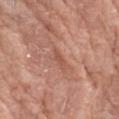Captured during whole-body skin photography for melanoma surveillance; the lesion was not biopsied. Automated tile analysis of the lesion measured a footprint of about 2.5 mm². It also reported about 7 CIELAB-L* units darker than the surrounding skin and a normalized lesion–skin contrast near 5.5. And it measured border irregularity of about 4.5 on a 0–10 scale, a within-lesion color-variation index near 0/10, and peripheral color asymmetry of about 0. It also reported an automated nevus-likeness rating near 0 out of 100. A female patient, about 75 years old. Measured at roughly 2.5 mm in maximum diameter. The tile uses white-light illumination. A lesion tile, about 15 mm wide, cut from a 3D total-body photograph. The lesion is located on the left forearm.